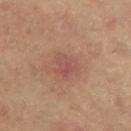• follow-up · no biopsy performed (imaged during a skin exam)
• site · the left thigh
• automated lesion analysis · a footprint of about 5.5 mm², an outline eccentricity of about 0.6 (0 = round, 1 = elongated), and a shape-asymmetry score of about 0.35 (0 = symmetric)
• illumination · cross-polarized illumination
• patient · female, aged 63–67
• lesion diameter · ~3 mm (longest diameter)
• imaging modality · total-body-photography crop, ~15 mm field of view